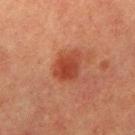{"biopsy_status": "not biopsied; imaged during a skin examination", "image": {"source": "total-body photography crop", "field_of_view_mm": 15}, "patient": {"sex": "female", "age_approx": 55}, "site": "arm", "lighting": "cross-polarized", "lesion_size": {"long_diameter_mm_approx": 3.5}, "automated_metrics": {"cielab_L": 36, "cielab_a": 27, "cielab_b": 29, "vs_skin_darker_L": 9.0, "vs_skin_contrast_norm": 8.0, "border_irregularity_0_10": 2.5, "peripheral_color_asymmetry": 1.0, "lesion_detection_confidence_0_100": 100}}